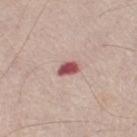workup = imaged on a skin check; not biopsied | location = the left thigh | subject = male, roughly 75 years of age | acquisition = 15 mm crop, total-body photography.